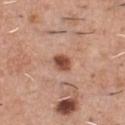<lesion>
  <biopsy_status>not biopsied; imaged during a skin examination</biopsy_status>
  <lesion_size>
    <long_diameter_mm_approx>2.5</long_diameter_mm_approx>
  </lesion_size>
  <site>chest</site>
  <patient>
    <sex>male</sex>
    <age_approx>45</age_approx>
  </patient>
  <automated_metrics>
    <area_mm2_approx>4.0</area_mm2_approx>
    <eccentricity>0.6</eccentricity>
    <shape_asymmetry>0.2</shape_asymmetry>
    <border_irregularity_0_10>2.0</border_irregularity_0_10>
    <color_variation_0_10>4.0</color_variation_0_10>
    <peripheral_color_asymmetry>1.5</peripheral_color_asymmetry>
    <nevus_likeness_0_100>90</nevus_likeness_0_100>
  </automated_metrics>
  <lighting>white-light</lighting>
  <image>
    <source>total-body photography crop</source>
    <field_of_view_mm>15</field_of_view_mm>
  </image>
</lesion>